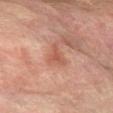Q: Was this lesion biopsied?
A: no biopsy performed (imaged during a skin exam)
Q: What is the anatomic site?
A: the arm
Q: What is the imaging modality?
A: total-body-photography crop, ~15 mm field of view
Q: Automated lesion metrics?
A: a mean CIELAB color near L≈44 a*≈21 b*≈26; a border-irregularity index near 5.5/10, a color-variation rating of about 1/10, and a peripheral color-asymmetry measure near 0; an automated nevus-likeness rating near 5 out of 100 and a detector confidence of about 100 out of 100 that the crop contains a lesion
Q: Patient demographics?
A: male, aged 73 to 77